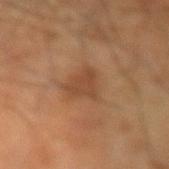| feature | finding |
|---|---|
| notes | imaged on a skin check; not biopsied |
| site | the right forearm |
| image source | ~15 mm tile from a whole-body skin photo |
| illumination | cross-polarized |
| image-analysis metrics | about 7 CIELAB-L* units darker than the surrounding skin; a border-irregularity index near 4/10, a color-variation rating of about 1.5/10, and a peripheral color-asymmetry measure near 0.5 |
| subject | male, aged around 60 |